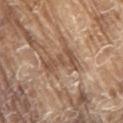Findings:
– follow-up: no biopsy performed (imaged during a skin exam)
– acquisition: 15 mm crop, total-body photography
– lighting: white-light illumination
– TBP lesion metrics: an area of roughly 8 mm², a shape eccentricity near 0.85, and two-axis asymmetry of about 0.55; a mean CIELAB color near L≈51 a*≈18 b*≈30, roughly 10 lightness units darker than nearby skin, and a normalized border contrast of about 7
– subject: male, approximately 80 years of age
– location: the right upper arm